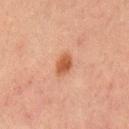Q: Is there a histopathology result?
A: imaged on a skin check; not biopsied
Q: Automated lesion metrics?
A: an automated nevus-likeness rating near 95 out of 100 and a detector confidence of about 100 out of 100 that the crop contains a lesion
Q: Where on the body is the lesion?
A: the mid back
Q: Who is the patient?
A: male, aged 63–67
Q: How was this image acquired?
A: ~15 mm tile from a whole-body skin photo